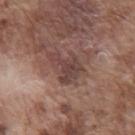Assessment:
Imaged during a routine full-body skin examination; the lesion was not biopsied and no histopathology is available.
Image and clinical context:
Located on the chest. A close-up tile cropped from a whole-body skin photograph, about 15 mm across. The subject is a male aged approximately 75. Automated image analysis of the tile measured a lesion area of about 9 mm², an outline eccentricity of about 0.75 (0 = round, 1 = elongated), and two-axis asymmetry of about 0.55. The software also gave a mean CIELAB color near L≈42 a*≈19 b*≈21 and roughly 9 lightness units darker than nearby skin. The software also gave a border-irregularity index near 7/10, internal color variation of about 2.5 on a 0–10 scale, and radial color variation of about 0.5. The software also gave a nevus-likeness score of about 5/100 and a detector confidence of about 100 out of 100 that the crop contains a lesion.